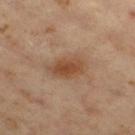Acquisition and patient details:
This image is a 15 mm lesion crop taken from a total-body photograph. About 4.5 mm across. On the right thigh. A male subject, aged around 60.